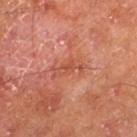  biopsy_status: not biopsied; imaged during a skin examination
  site: left thigh
  image:
    source: total-body photography crop
    field_of_view_mm: 15
  patient:
    sex: male
    age_approx: 65
  automated_metrics:
    area_mm2_approx: 5.0
    eccentricity: 0.9
    shape_asymmetry: 0.45